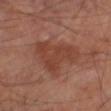Q: Was this lesion biopsied?
A: total-body-photography surveillance lesion; no biopsy